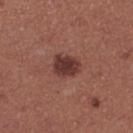No biopsy was performed on this lesion — it was imaged during a full skin examination and was not determined to be concerning. A female subject, aged 23–27. A 15 mm crop from a total-body photograph taken for skin-cancer surveillance. The lesion is on the leg.